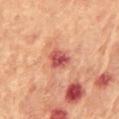biopsy status = catalogued during a skin exam; not biopsied
illumination = cross-polarized
image = total-body-photography crop, ~15 mm field of view
automated lesion analysis = a nevus-likeness score of about 0/100 and a detector confidence of about 100 out of 100 that the crop contains a lesion
body site = the chest
patient = female, aged around 80
lesion diameter = about 3 mm